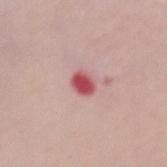| feature | finding |
|---|---|
| biopsy status | catalogued during a skin exam; not biopsied |
| site | the chest |
| automated metrics | a shape-asymmetry score of about 0.2 (0 = symmetric); border irregularity of about 1.5 on a 0–10 scale, a color-variation rating of about 2.5/10, and a peripheral color-asymmetry measure near 0.5; lesion-presence confidence of about 100/100 |
| lighting | white-light |
| size | ≈2.5 mm |
| patient | female, aged 63–67 |
| acquisition | total-body-photography crop, ~15 mm field of view |